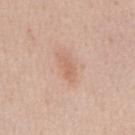Part of a total-body skin-imaging series; this lesion was reviewed on a skin check and was not flagged for biopsy.
On the mid back.
A male patient aged approximately 55.
This is a white-light tile.
A 15 mm crop from a total-body photograph taken for skin-cancer surveillance.